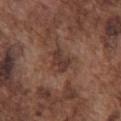Clinical impression: No biopsy was performed on this lesion — it was imaged during a full skin examination and was not determined to be concerning. Clinical summary: This image is a 15 mm lesion crop taken from a total-body photograph. A male subject in their mid-70s. The lesion-visualizer software estimated an area of roughly 5 mm², an outline eccentricity of about 0.75 (0 = round, 1 = elongated), and a shape-asymmetry score of about 0.3 (0 = symmetric). And it measured an average lesion color of about L≈36 a*≈19 b*≈23 (CIELAB), about 8 CIELAB-L* units darker than the surrounding skin, and a normalized lesion–skin contrast near 8. The software also gave a border-irregularity index near 3.5/10 and a color-variation rating of about 1.5/10. Located on the chest. The recorded lesion diameter is about 3 mm.